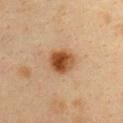Assessment: Part of a total-body skin-imaging series; this lesion was reviewed on a skin check and was not flagged for biopsy.